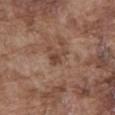follow-up: no biopsy performed (imaged during a skin exam); imaging modality: ~15 mm crop, total-body skin-cancer survey; site: the abdomen; patient: male, roughly 75 years of age; size: ≈2.5 mm; tile lighting: white-light illumination.